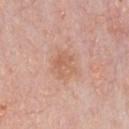Clinical impression:
This lesion was catalogued during total-body skin photography and was not selected for biopsy.
Context:
On the chest. A male patient, approximately 60 years of age. A close-up tile cropped from a whole-body skin photograph, about 15 mm across.